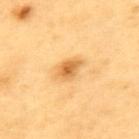Part of a total-body skin-imaging series; this lesion was reviewed on a skin check and was not flagged for biopsy. On the upper back. A region of skin cropped from a whole-body photographic capture, roughly 15 mm wide. A male subject, approximately 60 years of age.